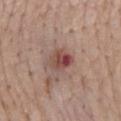notes = no biopsy performed (imaged during a skin exam) | site = the mid back | imaging modality = 15 mm crop, total-body photography | diameter = ≈3 mm | tile lighting = white-light illumination | patient = male, aged around 75.